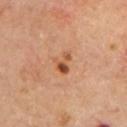biopsy status=total-body-photography surveillance lesion; no biopsy
lighting=cross-polarized illumination
lesion diameter=~3 mm (longest diameter)
automated metrics=border irregularity of about 6 on a 0–10 scale, a color-variation rating of about 3.5/10, and peripheral color asymmetry of about 1
site=the front of the torso
patient=male, aged approximately 65
image=total-body-photography crop, ~15 mm field of view